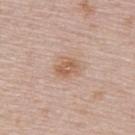workup=imaged on a skin check; not biopsied | imaging modality=15 mm crop, total-body photography | anatomic site=the upper back | patient=female, aged approximately 50.